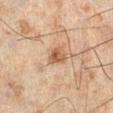Part of a total-body skin-imaging series; this lesion was reviewed on a skin check and was not flagged for biopsy. A male patient, roughly 45 years of age. A 15 mm crop from a total-body photograph taken for skin-cancer surveillance. Automated image analysis of the tile measured a lesion color around L≈45 a*≈17 b*≈29 in CIELAB and roughly 10 lightness units darker than nearby skin. It also reported lesion-presence confidence of about 100/100. The lesion is located on the left lower leg.